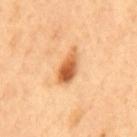Assessment: No biopsy was performed on this lesion — it was imaged during a full skin examination and was not determined to be concerning. Image and clinical context: Cropped from a total-body skin-imaging series; the visible field is about 15 mm. The lesion's longest dimension is about 4.5 mm. The subject is a male aged 48 to 52. The lesion is on the mid back. Automated image analysis of the tile measured a lesion area of about 7 mm² and a shape-asymmetry score of about 0.2 (0 = symmetric). It also reported an average lesion color of about L≈62 a*≈27 b*≈44 (CIELAB), about 16 CIELAB-L* units darker than the surrounding skin, and a normalized border contrast of about 10. Captured under cross-polarized illumination.